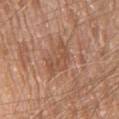<case>
<automated_metrics>
  <cielab_L>51</cielab_L>
  <cielab_a>21</cielab_a>
  <cielab_b>32</cielab_b>
  <vs_skin_contrast_norm>5.5</vs_skin_contrast_norm>
  <nevus_likeness_0_100>0</nevus_likeness_0_100>
  <lesion_detection_confidence_0_100>100</lesion_detection_confidence_0_100>
</automated_metrics>
<lesion_size>
  <long_diameter_mm_approx>4.5</long_diameter_mm_approx>
</lesion_size>
<patient>
  <sex>male</sex>
  <age_approx>80</age_approx>
</patient>
<image>
  <source>total-body photography crop</source>
  <field_of_view_mm>15</field_of_view_mm>
</image>
<site>chest</site>
<lighting>white-light</lighting>
</case>